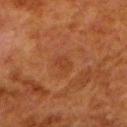biopsy status: no biopsy performed (imaged during a skin exam)
location: the left lower leg
imaging modality: total-body-photography crop, ~15 mm field of view
lesion size: ~2.5 mm (longest diameter)
subject: male, aged 78–82
tile lighting: cross-polarized